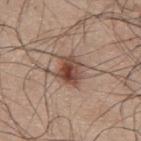notes: no biopsy performed (imaged during a skin exam)
image source: total-body-photography crop, ~15 mm field of view
TBP lesion metrics: a footprint of about 7.5 mm², an eccentricity of roughly 0.55, and a shape-asymmetry score of about 0.25 (0 = symmetric); an average lesion color of about L≈46 a*≈20 b*≈26 (CIELAB), a lesion–skin lightness drop of about 13, and a normalized border contrast of about 10; border irregularity of about 2.5 on a 0–10 scale and a color-variation rating of about 7.5/10
size: ≈3.5 mm
body site: the upper back
patient: male, in their mid- to late 40s